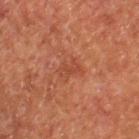Clinical impression: Captured during whole-body skin photography for melanoma surveillance; the lesion was not biopsied. Background: A close-up tile cropped from a whole-body skin photograph, about 15 mm across. A male patient, aged approximately 55. Approximately 3 mm at its widest. Located on the upper back. Captured under cross-polarized illumination.